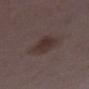Assessment: This lesion was catalogued during total-body skin photography and was not selected for biopsy. Clinical summary: Cropped from a total-body skin-imaging series; the visible field is about 15 mm. The lesion is located on the right lower leg. Approximately 4 mm at its widest. Automated tile analysis of the lesion measured an average lesion color of about L≈32 a*≈14 b*≈17 (CIELAB), about 7 CIELAB-L* units darker than the surrounding skin, and a lesion-to-skin contrast of about 7.5 (normalized; higher = more distinct). It also reported a nevus-likeness score of about 75/100 and a lesion-detection confidence of about 100/100. Imaged with white-light lighting. The patient is a female aged 28–32.